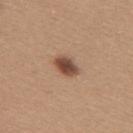Q: Is there a histopathology result?
A: imaged on a skin check; not biopsied
Q: Where on the body is the lesion?
A: the upper back
Q: What kind of image is this?
A: total-body-photography crop, ~15 mm field of view
Q: Patient demographics?
A: female, aged 28–32